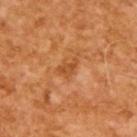The lesion was tiled from a total-body skin photograph and was not biopsied. This is a cross-polarized tile. The subject is a male about 65 years old. Automated image analysis of the tile measured peripheral color asymmetry of about 0.5. It also reported an automated nevus-likeness rating near 0 out of 100 and a detector confidence of about 100 out of 100 that the crop contains a lesion. Measured at roughly 2.5 mm in maximum diameter. A roughly 15 mm field-of-view crop from a total-body skin photograph.